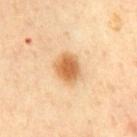Part of a total-body skin-imaging series; this lesion was reviewed on a skin check and was not flagged for biopsy. About 3.5 mm across. A male patient, aged around 60. Automated tile analysis of the lesion measured an area of roughly 8.5 mm², an eccentricity of roughly 0.6, and two-axis asymmetry of about 0.15. The analysis additionally found a mean CIELAB color near L≈63 a*≈22 b*≈42. The analysis additionally found border irregularity of about 1.5 on a 0–10 scale, internal color variation of about 4 on a 0–10 scale, and peripheral color asymmetry of about 1. Imaged with cross-polarized lighting. A roughly 15 mm field-of-view crop from a total-body skin photograph. The lesion is on the front of the torso.